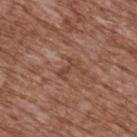  biopsy_status: not biopsied; imaged during a skin examination
  image:
    source: total-body photography crop
    field_of_view_mm: 15
  site: upper back
  patient:
    sex: male
    age_approx: 75
  automated_metrics:
    nevus_likeness_0_100: 0
    lesion_detection_confidence_0_100: 95
  lesion_size:
    long_diameter_mm_approx: 3.0